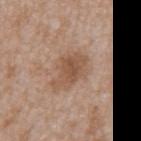Impression:
The lesion was photographed on a routine skin check and not biopsied; there is no pathology result.
Image and clinical context:
This is a white-light tile. A male patient in their mid- to late 60s. Cropped from a total-body skin-imaging series; the visible field is about 15 mm. The lesion is located on the back.